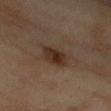Q: What is the lesion's diameter?
A: about 5 mm
Q: How was this image acquired?
A: 15 mm crop, total-body photography
Q: Lesion location?
A: the right upper arm
Q: Illumination type?
A: cross-polarized illumination
Q: Automated lesion metrics?
A: a lesion color around L≈29 a*≈14 b*≈23 in CIELAB and a lesion-to-skin contrast of about 8.5 (normalized; higher = more distinct)
Q: What are the patient's age and sex?
A: female, roughly 60 years of age